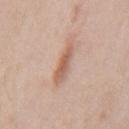This lesion was catalogued during total-body skin photography and was not selected for biopsy.
A male subject, in their mid- to late 60s.
The recorded lesion diameter is about 5 mm.
Located on the mid back.
Cropped from a whole-body photographic skin survey; the tile spans about 15 mm.
Automated image analysis of the tile measured a footprint of about 7 mm² and a symmetry-axis asymmetry near 0.2. The software also gave a mean CIELAB color near L≈61 a*≈20 b*≈29 and about 10 CIELAB-L* units darker than the surrounding skin. The analysis additionally found a border-irregularity rating of about 2.5/10, a color-variation rating of about 4/10, and radial color variation of about 1.5. The software also gave a nevus-likeness score of about 70/100 and a detector confidence of about 100 out of 100 that the crop contains a lesion.